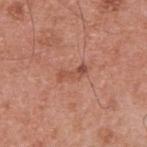notes = total-body-photography surveillance lesion; no biopsy
lesion diameter = ~3 mm (longest diameter)
image source = ~15 mm crop, total-body skin-cancer survey
image-analysis metrics = an eccentricity of roughly 0.95 and a symmetry-axis asymmetry near 0.45; a lesion color around L≈51 a*≈26 b*≈30 in CIELAB and roughly 9 lightness units darker than nearby skin
lighting = white-light illumination
subject = male, in their mid- to late 50s
body site = the upper back